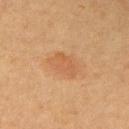Findings:
• biopsy status · catalogued during a skin exam; not biopsied
• lighting · cross-polarized
• TBP lesion metrics · an area of roughly 7 mm², an outline eccentricity of about 0.75 (0 = round, 1 = elongated), and a shape-asymmetry score of about 0.2 (0 = symmetric); an average lesion color of about L≈49 a*≈19 b*≈34 (CIELAB), roughly 6 lightness units darker than nearby skin, and a normalized lesion–skin contrast near 5
• patient · female, approximately 50 years of age
• image source · ~15 mm crop, total-body skin-cancer survey
• diameter · about 3.5 mm
• body site · the right upper arm